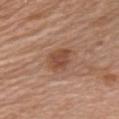<case>
<biopsy_status>not biopsied; imaged during a skin examination</biopsy_status>
<patient>
  <sex>male</sex>
  <age_approx>80</age_approx>
</patient>
<image>
  <source>total-body photography crop</source>
  <field_of_view_mm>15</field_of_view_mm>
</image>
<lesion_size>
  <long_diameter_mm_approx>3.5</long_diameter_mm_approx>
</lesion_size>
<lighting>white-light</lighting>
<site>chest</site>
<automated_metrics>
  <cielab_L>48</cielab_L>
  <cielab_a>22</cielab_a>
  <cielab_b>30</cielab_b>
  <vs_skin_darker_L>9.0</vs_skin_darker_L>
  <vs_skin_contrast_norm>7.0</vs_skin_contrast_norm>
  <border_irregularity_0_10>2.0</border_irregularity_0_10>
  <color_variation_0_10>3.0</color_variation_0_10>
  <peripheral_color_asymmetry>1.0</peripheral_color_asymmetry>
  <nevus_likeness_0_100>80</nevus_likeness_0_100>
  <lesion_detection_confidence_0_100>100</lesion_detection_confidence_0_100>
</automated_metrics>
</case>